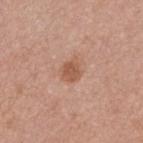workup = no biopsy performed (imaged during a skin exam)
diameter = ~2.5 mm (longest diameter)
body site = the right forearm
illumination = white-light
imaging modality = ~15 mm crop, total-body skin-cancer survey
subject = female, aged approximately 35